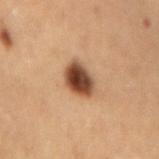Clinical impression: This lesion was catalogued during total-body skin photography and was not selected for biopsy. Image and clinical context: A female patient about 60 years old. The lesion is on the lower back. The recorded lesion diameter is about 3.5 mm. A 15 mm close-up tile from a total-body photography series done for melanoma screening.